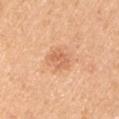Clinical impression: The lesion was tiled from a total-body skin photograph and was not biopsied. Context: On the arm. A region of skin cropped from a whole-body photographic capture, roughly 15 mm wide. The subject is a male aged approximately 50. Automated tile analysis of the lesion measured a within-lesion color-variation index near 3/10 and radial color variation of about 1.